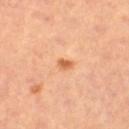notes: total-body-photography surveillance lesion; no biopsy | body site: the left thigh | patient: female, aged approximately 65 | lesion diameter: ~2 mm (longest diameter) | lighting: cross-polarized illumination | image source: total-body-photography crop, ~15 mm field of view.